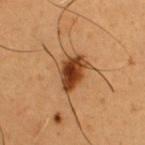image source: 15 mm crop, total-body photography
tile lighting: cross-polarized
TBP lesion metrics: a mean CIELAB color near L≈33 a*≈20 b*≈31 and about 14 CIELAB-L* units darker than the surrounding skin; a border-irregularity index near 2.5/10, a color-variation rating of about 5/10, and peripheral color asymmetry of about 1.5
body site: the chest
subject: male, aged around 50
lesion size: ~4.5 mm (longest diameter)